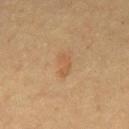The lesion was tiled from a total-body skin photograph and was not biopsied. A male subject, in their 60s. This is a cross-polarized tile. Automated image analysis of the tile measured a border-irregularity index near 3.5/10, internal color variation of about 2 on a 0–10 scale, and a peripheral color-asymmetry measure near 0.5. The lesion is located on the mid back. Approximately 3.5 mm at its widest. Cropped from a total-body skin-imaging series; the visible field is about 15 mm.